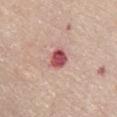The lesion was photographed on a routine skin check and not biopsied; there is no pathology result. This image is a 15 mm lesion crop taken from a total-body photograph. A female patient, in their 70s. Longest diameter approximately 3 mm. The lesion is located on the front of the torso. Automated image analysis of the tile measured a lesion area of about 5 mm², an eccentricity of roughly 0.7, and two-axis asymmetry of about 0.2. And it measured an average lesion color of about L≈52 a*≈33 b*≈22 (CIELAB), about 17 CIELAB-L* units darker than the surrounding skin, and a lesion-to-skin contrast of about 11.5 (normalized; higher = more distinct). The analysis additionally found a border-irregularity index near 1.5/10 and a peripheral color-asymmetry measure near 1.5. Imaged with white-light lighting.